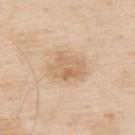Captured during whole-body skin photography for melanoma surveillance; the lesion was not biopsied. Located on the upper back. A roughly 15 mm field-of-view crop from a total-body skin photograph. A male subject, aged 78–82.